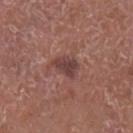notes: catalogued during a skin exam; not biopsied | location: the left lower leg | patient: male, aged around 65 | illumination: white-light | lesion diameter: about 3 mm | imaging modality: ~15 mm tile from a whole-body skin photo.